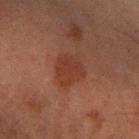{
  "patient": {
    "sex": "male",
    "age_approx": 60
  },
  "lighting": "cross-polarized",
  "site": "right forearm",
  "lesion_size": {
    "long_diameter_mm_approx": 4.0
  },
  "image": {
    "source": "total-body photography crop",
    "field_of_view_mm": 15
  }
}